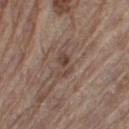Assessment: Imaged during a routine full-body skin examination; the lesion was not biopsied and no histopathology is available. Image and clinical context: The recorded lesion diameter is about 3 mm. A 15 mm close-up extracted from a 3D total-body photography capture. The total-body-photography lesion software estimated a lesion area of about 4 mm², a shape eccentricity near 0.85, and a symmetry-axis asymmetry near 0.55. The analysis additionally found a lesion color around L≈43 a*≈16 b*≈23 in CIELAB and a lesion-to-skin contrast of about 6.5 (normalized; higher = more distinct). On the left thigh. Captured under white-light illumination. The subject is a male approximately 65 years of age.